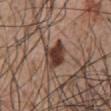Clinical impression: No biopsy was performed on this lesion — it was imaged during a full skin examination and was not determined to be concerning. Clinical summary: A close-up tile cropped from a whole-body skin photograph, about 15 mm across. Automated image analysis of the tile measured a lesion area of about 7.5 mm², an outline eccentricity of about 0.7 (0 = round, 1 = elongated), and a symmetry-axis asymmetry near 0.25. The software also gave a border-irregularity index near 2.5/10 and a within-lesion color-variation index near 5.5/10. A male patient, approximately 55 years of age. The lesion is on the chest. Imaged with white-light lighting. Measured at roughly 3.5 mm in maximum diameter.